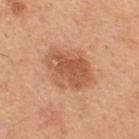Q: Was this lesion biopsied?
A: no biopsy performed (imaged during a skin exam)
Q: What is the anatomic site?
A: the upper back
Q: How was the tile lit?
A: white-light illumination
Q: Lesion size?
A: about 5.5 mm
Q: What are the patient's age and sex?
A: male, about 50 years old
Q: How was this image acquired?
A: total-body-photography crop, ~15 mm field of view